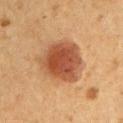Impression: Recorded during total-body skin imaging; not selected for excision or biopsy. Image and clinical context: A female patient, in their 40s. A lesion tile, about 15 mm wide, cut from a 3D total-body photograph. Imaged with cross-polarized lighting. The lesion is on the left upper arm.